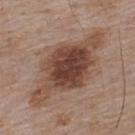Recorded during total-body skin imaging; not selected for excision or biopsy. This is a white-light tile. The patient is a male in their mid-50s. A lesion tile, about 15 mm wide, cut from a 3D total-body photograph. An algorithmic analysis of the crop reported an average lesion color of about L≈45 a*≈19 b*≈26 (CIELAB), about 13 CIELAB-L* units darker than the surrounding skin, and a lesion-to-skin contrast of about 10 (normalized; higher = more distinct). The software also gave border irregularity of about 4 on a 0–10 scale and internal color variation of about 6 on a 0–10 scale. And it measured a lesion-detection confidence of about 100/100. From the upper back. The recorded lesion diameter is about 11 mm.